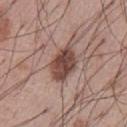Recorded during total-body skin imaging; not selected for excision or biopsy. On the abdomen. A close-up tile cropped from a whole-body skin photograph, about 15 mm across. This is a white-light tile. The subject is a male aged approximately 55. The lesion's longest dimension is about 4.5 mm. Automated image analysis of the tile measured a border-irregularity rating of about 1.5/10, a color-variation rating of about 4.5/10, and radial color variation of about 1.5.